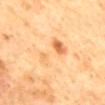lesion diameter: about 5.5 mm
image: ~15 mm tile from a whole-body skin photo
patient: female, roughly 65 years of age
image-analysis metrics: an outline eccentricity of about 0.9 (0 = round, 1 = elongated) and a shape-asymmetry score of about 0.4 (0 = symmetric); about 8 CIELAB-L* units darker than the surrounding skin; internal color variation of about 9.5 on a 0–10 scale and radial color variation of about 3
body site: the mid back